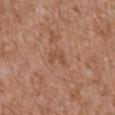<case>
<lighting>white-light</lighting>
<image>
  <source>total-body photography crop</source>
  <field_of_view_mm>15</field_of_view_mm>
</image>
<lesion_size>
  <long_diameter_mm_approx>2.5</long_diameter_mm_approx>
</lesion_size>
<site>front of the torso</site>
<patient>
  <sex>male</sex>
  <age_approx>75</age_approx>
</patient>
</case>